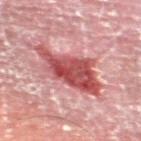| key | value |
|---|---|
| imaging modality | ~15 mm crop, total-body skin-cancer survey |
| location | the left thigh |
| tile lighting | cross-polarized illumination |
| TBP lesion metrics | border irregularity of about 4.5 on a 0–10 scale and radial color variation of about 2; lesion-presence confidence of about 80/100 |
| subject | male, in their mid- to late 50s |
| diameter | ≈8 mm |